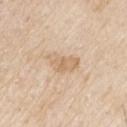Clinical impression:
The lesion was photographed on a routine skin check and not biopsied; there is no pathology result.
Acquisition and patient details:
The lesion is located on the right upper arm. A lesion tile, about 15 mm wide, cut from a 3D total-body photograph. The patient is a male roughly 80 years of age.